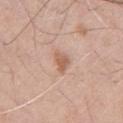<record>
<biopsy_status>not biopsied; imaged during a skin examination</biopsy_status>
<site>front of the torso</site>
<lesion_size>
  <long_diameter_mm_approx>2.5</long_diameter_mm_approx>
</lesion_size>
<patient>
  <sex>male</sex>
  <age_approx>70</age_approx>
</patient>
<image>
  <source>total-body photography crop</source>
  <field_of_view_mm>15</field_of_view_mm>
</image>
<lighting>white-light</lighting>
</record>